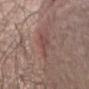Imaged during a routine full-body skin examination; the lesion was not biopsied and no histopathology is available. The recorded lesion diameter is about 3 mm. Cropped from a total-body skin-imaging series; the visible field is about 15 mm. The lesion is located on the abdomen. The subject is a male aged 63 to 67. Imaged with white-light lighting.